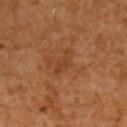biopsy status: no biopsy performed (imaged during a skin exam)
imaging modality: ~15 mm crop, total-body skin-cancer survey
patient: female, aged approximately 55
tile lighting: cross-polarized illumination
lesion size: ≈3.5 mm
location: the upper back